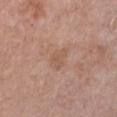• biopsy status: no biopsy performed (imaged during a skin exam)
• patient: female, in their 60s
• site: the chest
• image source: ~15 mm tile from a whole-body skin photo